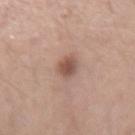workup = catalogued during a skin exam; not biopsied
image = total-body-photography crop, ~15 mm field of view
location = the right forearm
TBP lesion metrics = a border-irregularity index near 1.5/10, a within-lesion color-variation index near 3.5/10, and peripheral color asymmetry of about 1
patient = female, roughly 45 years of age
size = about 3 mm
lighting = white-light illumination